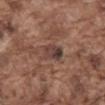biopsy status: catalogued during a skin exam; not biopsied | image source: total-body-photography crop, ~15 mm field of view | anatomic site: the abdomen | subject: male, aged approximately 75 | tile lighting: white-light.